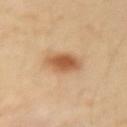Impression:
No biopsy was performed on this lesion — it was imaged during a full skin examination and was not determined to be concerning.
Context:
A female patient aged around 40. Automated tile analysis of the lesion measured a detector confidence of about 100 out of 100 that the crop contains a lesion. This is a cross-polarized tile. Longest diameter approximately 3.5 mm. The lesion is located on the left upper arm. Cropped from a total-body skin-imaging series; the visible field is about 15 mm.